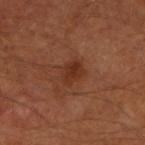Q: Was this lesion biopsied?
A: total-body-photography surveillance lesion; no biopsy
Q: How was this image acquired?
A: 15 mm crop, total-body photography
Q: What are the patient's age and sex?
A: male, roughly 60 years of age
Q: How was the tile lit?
A: cross-polarized
Q: Where on the body is the lesion?
A: the arm